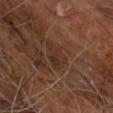The lesion was photographed on a routine skin check and not biopsied; there is no pathology result.
From the chest.
A male subject aged 58–62.
Automated tile analysis of the lesion measured an average lesion color of about L≈29 a*≈17 b*≈24 (CIELAB), about 5 CIELAB-L* units darker than the surrounding skin, and a normalized lesion–skin contrast near 5.5. And it measured a detector confidence of about 65 out of 100 that the crop contains a lesion.
The recorded lesion diameter is about 3.5 mm.
Cropped from a total-body skin-imaging series; the visible field is about 15 mm.
Imaged with cross-polarized lighting.